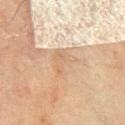{"biopsy_status": "not biopsied; imaged during a skin examination", "automated_metrics": {"area_mm2_approx": 6.5, "eccentricity": 0.45, "shape_asymmetry": 0.5, "border_irregularity_0_10": 5.5, "color_variation_0_10": 2.0}, "image": {"source": "total-body photography crop", "field_of_view_mm": 15}, "lesion_size": {"long_diameter_mm_approx": 3.5}, "patient": {"sex": "male", "age_approx": 70}, "site": "chest"}